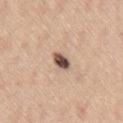Clinical summary: Longest diameter approximately 3 mm. The tile uses white-light illumination. Automated tile analysis of the lesion measured a symmetry-axis asymmetry near 0.2. The analysis additionally found a lesion color around L≈52 a*≈17 b*≈25 in CIELAB, roughly 20 lightness units darker than nearby skin, and a normalized border contrast of about 13. And it measured an automated nevus-likeness rating near 95 out of 100 and lesion-presence confidence of about 100/100. A 15 mm close-up tile from a total-body photography series done for melanoma screening. From the right thigh. A female patient roughly 45 years of age.